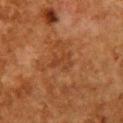Case summary:
- biopsy status · total-body-photography surveillance lesion; no biopsy
- image · 15 mm crop, total-body photography
- anatomic site · the chest
- subject · female, aged 48 to 52
- diameter · ~2.5 mm (longest diameter)